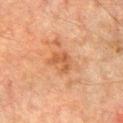The lesion was photographed on a routine skin check and not biopsied; there is no pathology result. A male patient, approximately 75 years of age. A region of skin cropped from a whole-body photographic capture, roughly 15 mm wide. From the front of the torso.